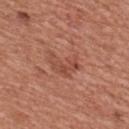Findings:
– workup · imaged on a skin check; not biopsied
– lesion size · ≈4 mm
– location · the front of the torso
– subject · male, aged 43–47
– acquisition · total-body-photography crop, ~15 mm field of view
– illumination · white-light illumination